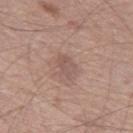Clinical impression: Imaged during a routine full-body skin examination; the lesion was not biopsied and no histopathology is available. Image and clinical context: Imaged with white-light lighting. On the left thigh. Approximately 3.5 mm at its widest. Cropped from a whole-body photographic skin survey; the tile spans about 15 mm. A male patient aged approximately 60.